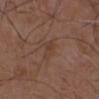Part of a total-body skin-imaging series; this lesion was reviewed on a skin check and was not flagged for biopsy. A male patient, aged 73–77. The tile uses white-light illumination. A 15 mm close-up tile from a total-body photography series done for melanoma screening. Approximately 3 mm at its widest. From the chest.